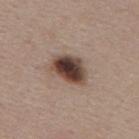Findings:
* follow-up: total-body-photography surveillance lesion; no biopsy
* lesion diameter: about 4.5 mm
* patient: female, aged around 45
* image source: ~15 mm crop, total-body skin-cancer survey
* image-analysis metrics: roughly 19 lightness units darker than nearby skin and a normalized lesion–skin contrast near 14; a border-irregularity rating of about 2/10 and a peripheral color-asymmetry measure near 2.5
* lighting: white-light illumination
* anatomic site: the mid back